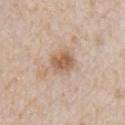Q: Is there a histopathology result?
A: no biopsy performed (imaged during a skin exam)
Q: Lesion size?
A: about 3 mm
Q: What did automated image analysis measure?
A: a footprint of about 6 mm² and an eccentricity of roughly 0.6; a border-irregularity index near 1.5/10 and a peripheral color-asymmetry measure near 1; a classifier nevus-likeness of about 40/100 and lesion-presence confidence of about 100/100
Q: Illumination type?
A: white-light illumination
Q: Who is the patient?
A: female, roughly 45 years of age
Q: What kind of image is this?
A: 15 mm crop, total-body photography
Q: What is the anatomic site?
A: the chest